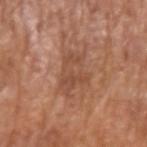No biopsy was performed on this lesion — it was imaged during a full skin examination and was not determined to be concerning. Measured at roughly 5.5 mm in maximum diameter. The subject is a male in their mid-70s. A roughly 15 mm field-of-view crop from a total-body skin photograph. This is a white-light tile. Located on the left upper arm.